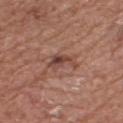The lesion was photographed on a routine skin check and not biopsied; there is no pathology result.
Imaged with white-light lighting.
The subject is a male in their mid-70s.
On the chest.
A roughly 15 mm field-of-view crop from a total-body skin photograph.
The recorded lesion diameter is about 3.5 mm.
The lesion-visualizer software estimated a footprint of about 5 mm², an outline eccentricity of about 0.85 (0 = round, 1 = elongated), and a shape-asymmetry score of about 0.45 (0 = symmetric). And it measured border irregularity of about 5.5 on a 0–10 scale, a color-variation rating of about 3.5/10, and peripheral color asymmetry of about 1.